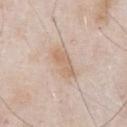<case>
<biopsy_status>not biopsied; imaged during a skin examination</biopsy_status>
<lesion_size>
  <long_diameter_mm_approx>4.0</long_diameter_mm_approx>
</lesion_size>
<image>
  <source>total-body photography crop</source>
  <field_of_view_mm>15</field_of_view_mm>
</image>
<patient>
  <sex>male</sex>
  <age_approx>55</age_approx>
</patient>
<automated_metrics>
  <area_mm2_approx>7.0</area_mm2_approx>
  <shape_asymmetry>0.2</shape_asymmetry>
  <vs_skin_darker_L>8.0</vs_skin_darker_L>
  <vs_skin_contrast_norm>6.0</vs_skin_contrast_norm>
</automated_metrics>
<lighting>white-light</lighting>
<site>chest</site>
</case>